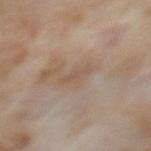The lesion was tiled from a total-body skin photograph and was not biopsied.
A 15 mm crop from a total-body photograph taken for skin-cancer surveillance.
A female subject, roughly 60 years of age.
On the back.
About 3 mm across.